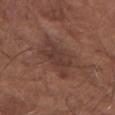Part of a total-body skin-imaging series; this lesion was reviewed on a skin check and was not flagged for biopsy. Imaged with white-light lighting. Located on the right forearm. About 6 mm across. This image is a 15 mm lesion crop taken from a total-body photograph. Automated image analysis of the tile measured a lesion color around L≈37 a*≈19 b*≈23 in CIELAB, roughly 7 lightness units darker than nearby skin, and a normalized border contrast of about 6.5. And it measured a lesion-detection confidence of about 95/100. A male patient, in their mid- to late 70s.